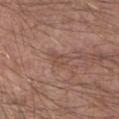Imaged during a routine full-body skin examination; the lesion was not biopsied and no histopathology is available.
A region of skin cropped from a whole-body photographic capture, roughly 15 mm wide.
This is a white-light tile.
A male subject, in their mid-50s.
The lesion is on the arm.
The total-body-photography lesion software estimated a color-variation rating of about 2.5/10 and radial color variation of about 1.